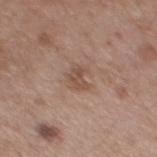Clinical impression: Recorded during total-body skin imaging; not selected for excision or biopsy. Clinical summary: The subject is a male about 55 years old. The lesion is located on the mid back. Captured under white-light illumination. A lesion tile, about 15 mm wide, cut from a 3D total-body photograph.